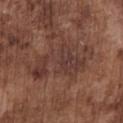workup: total-body-photography surveillance lesion; no biopsy
patient: male, in their mid- to late 70s
tile lighting: white-light
automated lesion analysis: roughly 7 lightness units darker than nearby skin and a normalized border contrast of about 6.5; lesion-presence confidence of about 70/100
body site: the chest
image: 15 mm crop, total-body photography
size: about 8.5 mm